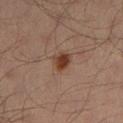follow-up — no biopsy performed (imaged during a skin exam)
tile lighting — cross-polarized illumination
image source — 15 mm crop, total-body photography
body site — the left lower leg
TBP lesion metrics — an outline eccentricity of about 0.45 (0 = round, 1 = elongated); a border-irregularity index near 1.5/10, a within-lesion color-variation index near 4.5/10, and a peripheral color-asymmetry measure near 1; an automated nevus-likeness rating near 100 out of 100 and a detector confidence of about 100 out of 100 that the crop contains a lesion
subject — male, about 35 years old
diameter — ≈2.5 mm